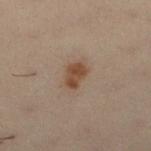| key | value |
|---|---|
| notes | catalogued during a skin exam; not biopsied |
| acquisition | ~15 mm crop, total-body skin-cancer survey |
| site | the left leg |
| subject | female, approximately 30 years of age |